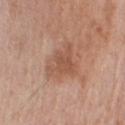notes — total-body-photography surveillance lesion; no biopsy
body site — the right upper arm
imaging modality — 15 mm crop, total-body photography
diameter — about 4.5 mm
image-analysis metrics — an area of roughly 12 mm², a shape eccentricity near 0.5, and a symmetry-axis asymmetry near 0.4; a mean CIELAB color near L≈54 a*≈21 b*≈30, roughly 8 lightness units darker than nearby skin, and a normalized border contrast of about 6; a border-irregularity rating of about 4/10, a within-lesion color-variation index near 4.5/10, and peripheral color asymmetry of about 1.5
subject — male, aged around 55